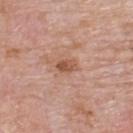A roughly 15 mm field-of-view crop from a total-body skin photograph. Located on the upper back. A male subject aged approximately 75. Longest diameter approximately 2.5 mm.